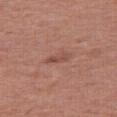{"biopsy_status": "not biopsied; imaged during a skin examination", "site": "leg", "lighting": "white-light", "lesion_size": {"long_diameter_mm_approx": 2.5}, "image": {"source": "total-body photography crop", "field_of_view_mm": 15}, "patient": {"sex": "female", "age_approx": 40}}